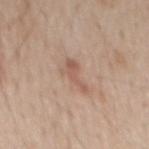The patient is a male aged approximately 60. A 15 mm close-up extracted from a 3D total-body photography capture. Automated image analysis of the tile measured a classifier nevus-likeness of about 0/100. Measured at roughly 4 mm in maximum diameter. The lesion is located on the mid back.